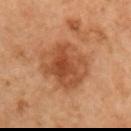  biopsy_status: not biopsied; imaged during a skin examination
  patient:
    sex: male
    age_approx: 70
  lighting: cross-polarized
  automated_metrics:
    area_mm2_approx: 24.0
    eccentricity: 0.15
    shape_asymmetry: 0.15
    border_irregularity_0_10: 2.0
    peripheral_color_asymmetry: 1.5
    lesion_detection_confidence_0_100: 100
  image:
    source: total-body photography crop
    field_of_view_mm: 15
  site: right upper arm